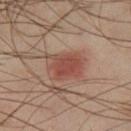biopsy status = imaged on a skin check; not biopsied | tile lighting = cross-polarized | acquisition = ~15 mm tile from a whole-body skin photo | patient = male, aged 38–42 | automated metrics = a border-irregularity index near 2.5/10, internal color variation of about 4 on a 0–10 scale, and radial color variation of about 1.5; an automated nevus-likeness rating near 100 out of 100 and lesion-presence confidence of about 100/100 | location = the right thigh | diameter = about 4.5 mm.